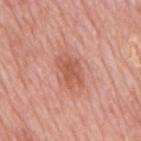– follow-up · imaged on a skin check; not biopsied
– diameter · ~4 mm (longest diameter)
– patient · male, in their mid- to late 60s
– illumination · white-light
– imaging modality · total-body-photography crop, ~15 mm field of view
– site · the back
– TBP lesion metrics · an area of roughly 7.5 mm², an eccentricity of roughly 0.8, and a shape-asymmetry score of about 0.2 (0 = symmetric); a detector confidence of about 100 out of 100 that the crop contains a lesion